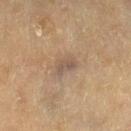workup=no biopsy performed (imaged during a skin exam)
subject=female, approximately 80 years of age
body site=the leg
acquisition=15 mm crop, total-body photography
tile lighting=cross-polarized illumination
diameter=≈3 mm